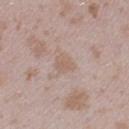Part of a total-body skin-imaging series; this lesion was reviewed on a skin check and was not flagged for biopsy. Measured at roughly 2.5 mm in maximum diameter. On the right lower leg. A female subject, approximately 25 years of age. An algorithmic analysis of the crop reported a lesion color around L≈60 a*≈16 b*≈25 in CIELAB. And it measured a detector confidence of about 100 out of 100 that the crop contains a lesion. A region of skin cropped from a whole-body photographic capture, roughly 15 mm wide. The tile uses white-light illumination.